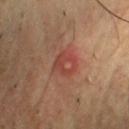Clinical impression:
Part of a total-body skin-imaging series; this lesion was reviewed on a skin check and was not flagged for biopsy.
Acquisition and patient details:
A region of skin cropped from a whole-body photographic capture, roughly 15 mm wide. Automated image analysis of the tile measured a lesion area of about 6.5 mm², an eccentricity of roughly 0.25, and a shape-asymmetry score of about 0.2 (0 = symmetric). The software also gave a mean CIELAB color near L≈42 a*≈26 b*≈27, roughly 6 lightness units darker than nearby skin, and a lesion-to-skin contrast of about 5.5 (normalized; higher = more distinct). Captured under cross-polarized illumination. From the front of the torso. A male subject, in their 50s. About 3 mm across.